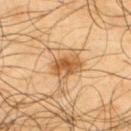Clinical summary:
A male patient, roughly 65 years of age. This is a cross-polarized tile. Located on the upper back. The total-body-photography lesion software estimated a lesion area of about 13 mm², an outline eccentricity of about 0.7 (0 = round, 1 = elongated), and two-axis asymmetry of about 0.45. The software also gave an average lesion color of about L≈57 a*≈19 b*≈39 (CIELAB), a lesion–skin lightness drop of about 11, and a normalized lesion–skin contrast near 7.5. It also reported internal color variation of about 7.5 on a 0–10 scale and peripheral color asymmetry of about 2. Approximately 5.5 mm at its widest. Cropped from a whole-body photographic skin survey; the tile spans about 15 mm.